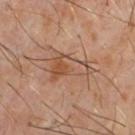Longest diameter approximately 4.5 mm. A 15 mm crop from a total-body photograph taken for skin-cancer surveillance. A male patient aged around 60. Automated tile analysis of the lesion measured a detector confidence of about 100 out of 100 that the crop contains a lesion. The lesion is located on the chest. The tile uses cross-polarized illumination.